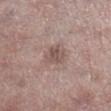Assessment:
No biopsy was performed on this lesion — it was imaged during a full skin examination and was not determined to be concerning.
Acquisition and patient details:
The lesion is located on the leg. Captured under white-light illumination. The patient is a male approximately 55 years of age. A 15 mm crop from a total-body photograph taken for skin-cancer surveillance. The lesion-visualizer software estimated an area of roughly 6 mm² and an outline eccentricity of about 0.2 (0 = round, 1 = elongated). And it measured a lesion–skin lightness drop of about 9 and a normalized border contrast of about 6.5. The software also gave a detector confidence of about 100 out of 100 that the crop contains a lesion.